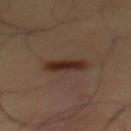biopsy_status: not biopsied; imaged during a skin examination
patient:
  sex: male
  age_approx: 55
lighting: cross-polarized
site: front of the torso
automated_metrics:
  area_mm2_approx: 7.0
  eccentricity: 0.9
  shape_asymmetry: 0.25
  border_irregularity_0_10: 3.0
  peripheral_color_asymmetry: 1.0
  nevus_likeness_0_100: 100
  lesion_detection_confidence_0_100: 100
image:
  source: total-body photography crop
  field_of_view_mm: 15